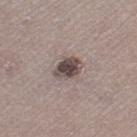Part of a total-body skin-imaging series; this lesion was reviewed on a skin check and was not flagged for biopsy. A female patient, roughly 50 years of age. Measured at roughly 3.5 mm in maximum diameter. A close-up tile cropped from a whole-body skin photograph, about 15 mm across. On the left thigh.